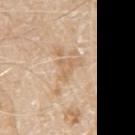The lesion was tiled from a total-body skin photograph and was not biopsied. The patient is a male aged approximately 80. A 15 mm crop from a total-body photograph taken for skin-cancer surveillance. The tile uses white-light illumination. Located on the left upper arm. The lesion's longest dimension is about 3.5 mm.